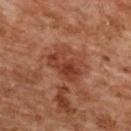biopsy status: total-body-photography surveillance lesion; no biopsy
location: the upper back
lighting: cross-polarized
patient: female, aged around 60
image source: ~15 mm crop, total-body skin-cancer survey